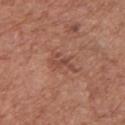Assessment:
This lesion was catalogued during total-body skin photography and was not selected for biopsy.
Clinical summary:
A 15 mm crop from a total-body photograph taken for skin-cancer surveillance. This is a white-light tile. A male subject aged 73 to 77. On the chest. About 3.5 mm across.